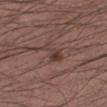Q: Was this lesion biopsied?
A: imaged on a skin check; not biopsied
Q: What is the imaging modality?
A: total-body-photography crop, ~15 mm field of view
Q: Patient demographics?
A: male, aged approximately 40
Q: Lesion location?
A: the leg
Q: Lesion size?
A: ≈3.5 mm
Q: Illumination type?
A: white-light illumination